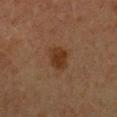biopsy status — no biopsy performed (imaged during a skin exam) | acquisition — total-body-photography crop, ~15 mm field of view | tile lighting — cross-polarized illumination | body site — the chest | automated metrics — a lesion area of about 6 mm² and an outline eccentricity of about 0.65 (0 = round, 1 = elongated); a normalized border contrast of about 9; border irregularity of about 2 on a 0–10 scale, a color-variation rating of about 1.5/10, and peripheral color asymmetry of about 0.5 | patient — female, approximately 50 years of age | lesion diameter — ≈3 mm.